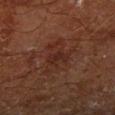Recorded during total-body skin imaging; not selected for excision or biopsy. A male subject, approximately 65 years of age. The tile uses cross-polarized illumination. Automated tile analysis of the lesion measured a symmetry-axis asymmetry near 0.5. The software also gave a color-variation rating of about 4.5/10 and peripheral color asymmetry of about 1.5. This image is a 15 mm lesion crop taken from a total-body photograph. The lesion is located on the right lower leg. Longest diameter approximately 5.5 mm.